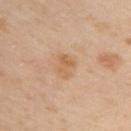Recorded during total-body skin imaging; not selected for excision or biopsy. About 3 mm across. The lesion-visualizer software estimated an area of roughly 5 mm², a shape eccentricity near 0.65, and a symmetry-axis asymmetry near 0.25. The software also gave a lesion–skin lightness drop of about 7 and a normalized lesion–skin contrast near 5.5. A 15 mm crop from a total-body photograph taken for skin-cancer surveillance. The tile uses cross-polarized illumination. A female subject aged 38–42. The lesion is on the upper back.